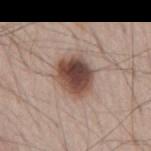Notes:
* follow-up — catalogued during a skin exam; not biopsied
* tile lighting — white-light illumination
* automated metrics — a lesion area of about 14 mm² and an outline eccentricity of about 0.55 (0 = round, 1 = elongated); a nevus-likeness score of about 95/100 and a lesion-detection confidence of about 100/100
* lesion size — ~4.5 mm (longest diameter)
* image source — total-body-photography crop, ~15 mm field of view
* patient — male, in their mid- to late 60s
* body site — the mid back